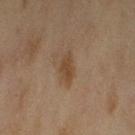Part of a total-body skin-imaging series; this lesion was reviewed on a skin check and was not flagged for biopsy.
A lesion tile, about 15 mm wide, cut from a 3D total-body photograph.
A female subject, approximately 60 years of age.
Located on the left upper arm.
The tile uses cross-polarized illumination.
About 3 mm across.